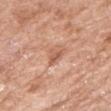Part of a total-body skin-imaging series; this lesion was reviewed on a skin check and was not flagged for biopsy. Cropped from a whole-body photographic skin survey; the tile spans about 15 mm. The subject is a female in their mid- to late 70s. The recorded lesion diameter is about 2.5 mm. On the right upper arm.